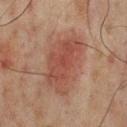This lesion was catalogued during total-body skin photography and was not selected for biopsy.
Automated image analysis of the tile measured a mean CIELAB color near L≈38 a*≈20 b*≈23, roughly 7 lightness units darker than nearby skin, and a normalized lesion–skin contrast near 6.5. And it measured a lesion-detection confidence of about 100/100.
Cropped from a total-body skin-imaging series; the visible field is about 15 mm.
The subject is a male aged approximately 65.
The lesion's longest dimension is about 7 mm.
This is a cross-polarized tile.
On the mid back.